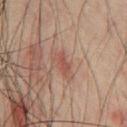Assessment: Imaged during a routine full-body skin examination; the lesion was not biopsied and no histopathology is available. Acquisition and patient details: On the chest. Longest diameter approximately 2.5 mm. The tile uses cross-polarized illumination. A 15 mm close-up tile from a total-body photography series done for melanoma screening. The subject is a male roughly 60 years of age.